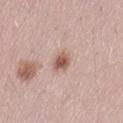Clinical impression: The lesion was tiled from a total-body skin photograph and was not biopsied. Acquisition and patient details: The lesion is located on the abdomen. The total-body-photography lesion software estimated a border-irregularity index near 2/10 and internal color variation of about 3.5 on a 0–10 scale. The analysis additionally found an automated nevus-likeness rating near 90 out of 100 and a detector confidence of about 100 out of 100 that the crop contains a lesion. The lesion's longest dimension is about 2.5 mm. A close-up tile cropped from a whole-body skin photograph, about 15 mm across. This is a white-light tile. The subject is a female aged 23 to 27.